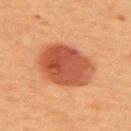Part of a total-body skin-imaging series; this lesion was reviewed on a skin check and was not flagged for biopsy. The lesion is on the upper back. Automated tile analysis of the lesion measured an automated nevus-likeness rating near 100 out of 100 and a detector confidence of about 100 out of 100 that the crop contains a lesion. A female subject aged approximately 50. A 15 mm close-up tile from a total-body photography series done for melanoma screening. Imaged with cross-polarized lighting. The recorded lesion diameter is about 7 mm.